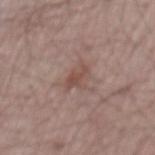<record>
<biopsy_status>not biopsied; imaged during a skin examination</biopsy_status>
<site>mid back</site>
<image>
  <source>total-body photography crop</source>
  <field_of_view_mm>15</field_of_view_mm>
</image>
<patient>
  <sex>male</sex>
  <age_approx>65</age_approx>
</patient>
</record>